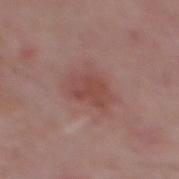Notes:
• biopsy status · total-body-photography surveillance lesion; no biopsy
• patient · male, aged approximately 65
• anatomic site · the back
• tile lighting · white-light
• automated lesion analysis · an outline eccentricity of about 0.65 (0 = round, 1 = elongated) and a shape-asymmetry score of about 0.3 (0 = symmetric); a lesion color around L≈47 a*≈23 b*≈23 in CIELAB, a lesion–skin lightness drop of about 7, and a normalized border contrast of about 6; a border-irregularity rating of about 3.5/10 and a color-variation rating of about 3/10
• imaging modality · ~15 mm crop, total-body skin-cancer survey
• size · ~4.5 mm (longest diameter)